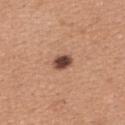Q: Was a biopsy performed?
A: catalogued during a skin exam; not biopsied
Q: Illumination type?
A: white-light
Q: How large is the lesion?
A: ~2.5 mm (longest diameter)
Q: Patient demographics?
A: female, roughly 30 years of age
Q: What is the imaging modality?
A: 15 mm crop, total-body photography
Q: Where on the body is the lesion?
A: the back